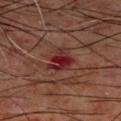Clinical impression:
The lesion was photographed on a routine skin check and not biopsied; there is no pathology result.
Context:
The patient is a male approximately 60 years of age. On the upper back. Automated tile analysis of the lesion measured a mean CIELAB color near L≈25 a*≈27 b*≈21, a lesion–skin lightness drop of about 9, and a normalized lesion–skin contrast near 9.5. The analysis additionally found a border-irregularity rating of about 4.5/10 and a peripheral color-asymmetry measure near 1.5. And it measured a nevus-likeness score of about 0/100 and a lesion-detection confidence of about 100/100. Cropped from a total-body skin-imaging series; the visible field is about 15 mm. Measured at roughly 3.5 mm in maximum diameter. Imaged with cross-polarized lighting.